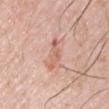{"biopsy_status": "not biopsied; imaged during a skin examination", "image": {"source": "total-body photography crop", "field_of_view_mm": 15}, "patient": {"sex": "male", "age_approx": 65}, "lighting": "white-light", "site": "right upper arm"}